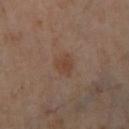* subject · female, about 55 years old
* image source · ~15 mm tile from a whole-body skin photo
* site · the leg